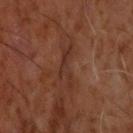Q: Is there a histopathology result?
A: catalogued during a skin exam; not biopsied
Q: Automated lesion metrics?
A: a lesion color around L≈29 a*≈20 b*≈25 in CIELAB, a lesion–skin lightness drop of about 6, and a normalized border contrast of about 6; a border-irregularity index near 8.5/10, internal color variation of about 0 on a 0–10 scale, and radial color variation of about 0; a classifier nevus-likeness of about 0/100 and lesion-presence confidence of about 55/100
Q: What lighting was used for the tile?
A: cross-polarized
Q: Where on the body is the lesion?
A: the head or neck
Q: What kind of image is this?
A: total-body-photography crop, ~15 mm field of view
Q: How large is the lesion?
A: ≈5.5 mm
Q: What are the patient's age and sex?
A: male, roughly 60 years of age